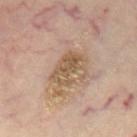The lesion was photographed on a routine skin check and not biopsied; there is no pathology result.
The total-body-photography lesion software estimated a footprint of about 10 mm² and a symmetry-axis asymmetry near 0.3. And it measured an average lesion color of about L≈54 a*≈15 b*≈31 (CIELAB), a lesion–skin lightness drop of about 9, and a lesion-to-skin contrast of about 7 (normalized; higher = more distinct). The analysis additionally found a classifier nevus-likeness of about 0/100.
The lesion's longest dimension is about 4.5 mm.
This is a cross-polarized tile.
The patient is a female in their mid- to late 40s.
Located on the mid back.
A lesion tile, about 15 mm wide, cut from a 3D total-body photograph.